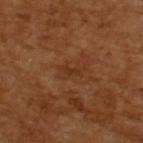Imaged during a routine full-body skin examination; the lesion was not biopsied and no histopathology is available. Automated tile analysis of the lesion measured a shape eccentricity near 0.85 and two-axis asymmetry of about 0.35. It also reported a mean CIELAB color near L≈33 a*≈22 b*≈32, roughly 5 lightness units darker than nearby skin, and a lesion-to-skin contrast of about 4.5 (normalized; higher = more distinct). The software also gave a nevus-likeness score of about 0/100 and a lesion-detection confidence of about 100/100. The lesion's longest dimension is about 3 mm. The patient is a male about 65 years old. A 15 mm crop from a total-body photograph taken for skin-cancer surveillance. Captured under cross-polarized illumination.